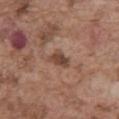<tbp_lesion>
  <biopsy_status>not biopsied; imaged during a skin examination</biopsy_status>
  <automated_metrics>
    <area_mm2_approx>4.0</area_mm2_approx>
    <eccentricity>0.75</eccentricity>
    <shape_asymmetry>0.3</shape_asymmetry>
    <color_variation_0_10>2.0</color_variation_0_10>
    <peripheral_color_asymmetry>0.5</peripheral_color_asymmetry>
  </automated_metrics>
  <image>
    <source>total-body photography crop</source>
    <field_of_view_mm>15</field_of_view_mm>
  </image>
  <site>abdomen</site>
  <patient>
    <sex>male</sex>
    <age_approx>75</age_approx>
  </patient>
  <lighting>white-light</lighting>
</tbp_lesion>